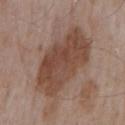Case summary:
* automated metrics: a peripheral color-asymmetry measure near 1.5
* anatomic site: the arm
* tile lighting: white-light
* image source: ~15 mm crop, total-body skin-cancer survey
* subject: male, in their mid-50s